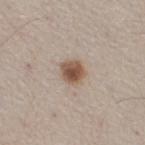<case>
  <biopsy_status>not biopsied; imaged during a skin examination</biopsy_status>
</case>